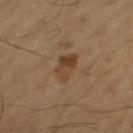Clinical impression:
Imaged during a routine full-body skin examination; the lesion was not biopsied and no histopathology is available.
Clinical summary:
A male subject approximately 65 years of age. A 15 mm close-up extracted from a 3D total-body photography capture. Located on the left thigh.